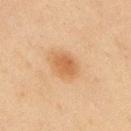<case>
<biopsy_status>not biopsied; imaged during a skin examination</biopsy_status>
<patient>
  <sex>male</sex>
  <age_approx>45</age_approx>
</patient>
<site>upper back</site>
<automated_metrics>
  <cielab_L>49</cielab_L>
  <cielab_a>19</cielab_a>
  <cielab_b>34</cielab_b>
  <vs_skin_darker_L>8.0</vs_skin_darker_L>
  <vs_skin_contrast_norm>7.0</vs_skin_contrast_norm>
  <border_irregularity_0_10>2.0</border_irregularity_0_10>
  <color_variation_0_10>2.0</color_variation_0_10>
</automated_metrics>
<lesion_size>
  <long_diameter_mm_approx>3.5</long_diameter_mm_approx>
</lesion_size>
<lighting>cross-polarized</lighting>
<image>
  <source>total-body photography crop</source>
  <field_of_view_mm>15</field_of_view_mm>
</image>
</case>